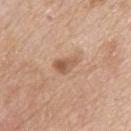No biopsy was performed on this lesion — it was imaged during a full skin examination and was not determined to be concerning. A roughly 15 mm field-of-view crop from a total-body skin photograph. Captured under white-light illumination. The recorded lesion diameter is about 3 mm. Automated tile analysis of the lesion measured a footprint of about 4 mm² and two-axis asymmetry of about 0.4. The software also gave a border-irregularity rating of about 3.5/10. And it measured an automated nevus-likeness rating near 55 out of 100 and lesion-presence confidence of about 100/100. The patient is a male roughly 80 years of age. Located on the back.